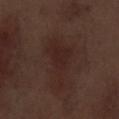Findings:
• biopsy status — catalogued during a skin exam; not biopsied
• image-analysis metrics — a shape eccentricity near 0.85 and a symmetry-axis asymmetry near 0.55
• imaging modality — ~15 mm crop, total-body skin-cancer survey
• illumination — white-light illumination
• lesion diameter — about 6.5 mm
• patient — male, roughly 70 years of age
• body site — the left thigh